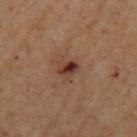A male subject, aged 68–72. The tile uses cross-polarized illumination. The lesion is on the left upper arm. A 15 mm close-up extracted from a 3D total-body photography capture.